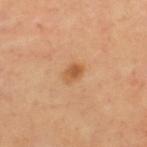This lesion was catalogued during total-body skin photography and was not selected for biopsy.
On the upper back.
An algorithmic analysis of the crop reported an average lesion color of about L≈56 a*≈23 b*≈39 (CIELAB) and a lesion-to-skin contrast of about 7 (normalized; higher = more distinct).
This image is a 15 mm lesion crop taken from a total-body photograph.
This is a cross-polarized tile.
Measured at roughly 2.5 mm in maximum diameter.
A male patient aged 53 to 57.